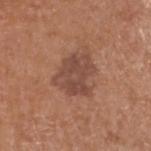Q: Is there a histopathology result?
A: total-body-photography surveillance lesion; no biopsy